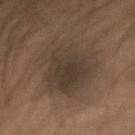Imaged during a routine full-body skin examination; the lesion was not biopsied and no histopathology is available.
From the right forearm.
The subject is a male about 40 years old.
A region of skin cropped from a whole-body photographic capture, roughly 15 mm wide.
The tile uses cross-polarized illumination.
About 9 mm across.